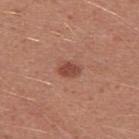tile lighting — white-light illumination | subject — male, approximately 25 years of age | image — ~15 mm tile from a whole-body skin photo | site — the right upper arm.